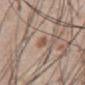workup: catalogued during a skin exam; not biopsied | anatomic site: the abdomen | patient: male, aged 58–62 | image: ~15 mm tile from a whole-body skin photo.